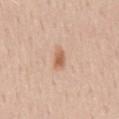biopsy status: total-body-photography surveillance lesion; no biopsy | body site: the lower back | image source: ~15 mm crop, total-body skin-cancer survey | automated metrics: a lesion color around L≈63 a*≈21 b*≈33 in CIELAB, a lesion–skin lightness drop of about 10, and a normalized lesion–skin contrast near 7.5; a border-irregularity index near 2/10, a within-lesion color-variation index near 2.5/10, and peripheral color asymmetry of about 0.5; an automated nevus-likeness rating near 95 out of 100 and lesion-presence confidence of about 100/100 | illumination: white-light | subject: male, aged 58–62.